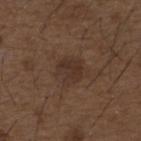  biopsy_status: not biopsied; imaged during a skin examination
  lighting: white-light
  lesion_size:
    long_diameter_mm_approx: 3.5
  image:
    source: total-body photography crop
    field_of_view_mm: 15
  patient:
    sex: male
    age_approx: 50
  site: upper back